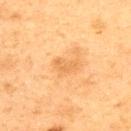Q: Was a biopsy performed?
A: imaged on a skin check; not biopsied
Q: Patient demographics?
A: male, about 75 years old
Q: What kind of image is this?
A: 15 mm crop, total-body photography
Q: Where on the body is the lesion?
A: the upper back